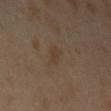Q: Was this lesion biopsied?
A: no biopsy performed (imaged during a skin exam)
Q: What is the lesion's diameter?
A: about 3 mm
Q: What kind of image is this?
A: ~15 mm tile from a whole-body skin photo
Q: Patient demographics?
A: female, in their 40s
Q: Where on the body is the lesion?
A: the arm
Q: What did automated image analysis measure?
A: a footprint of about 3.5 mm² and a shape eccentricity near 0.8; a border-irregularity index near 3.5/10, a within-lesion color-variation index near 1/10, and peripheral color asymmetry of about 0.5
Q: What lighting was used for the tile?
A: cross-polarized